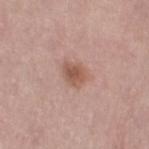<tbp_lesion>
<image>
  <source>total-body photography crop</source>
  <field_of_view_mm>15</field_of_view_mm>
</image>
<lesion_size>
  <long_diameter_mm_approx>3.0</long_diameter_mm_approx>
</lesion_size>
<lighting>white-light</lighting>
<patient>
  <sex>female</sex>
  <age_approx>65</age_approx>
</patient>
<site>left thigh</site>
</tbp_lesion>